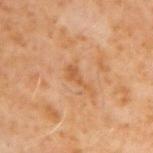{
  "biopsy_status": "not biopsied; imaged during a skin examination"
}